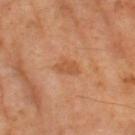Recorded during total-body skin imaging; not selected for excision or biopsy. The tile uses cross-polarized illumination. The lesion's longest dimension is about 3 mm. The subject is a male aged approximately 65. This image is a 15 mm lesion crop taken from a total-body photograph. The total-body-photography lesion software estimated an eccentricity of roughly 0.8 and two-axis asymmetry of about 0.3. The analysis additionally found a within-lesion color-variation index near 1.5/10 and radial color variation of about 0.5.